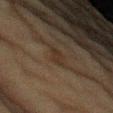{"image": {"source": "total-body photography crop", "field_of_view_mm": 15}, "patient": {"sex": "male", "age_approx": 75}, "site": "left upper arm", "lesion_size": {"long_diameter_mm_approx": 2.5}, "lighting": "cross-polarized"}